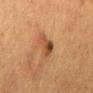Background:
The tile uses cross-polarized illumination. This image is a 15 mm lesion crop taken from a total-body photograph. An algorithmic analysis of the crop reported a lesion area of about 5.5 mm², a shape eccentricity near 0.45, and two-axis asymmetry of about 0.45. It also reported an average lesion color of about L≈39 a*≈20 b*≈30 (CIELAB), a lesion–skin lightness drop of about 10, and a lesion-to-skin contrast of about 8.5 (normalized; higher = more distinct). And it measured a nevus-likeness score of about 85/100 and lesion-presence confidence of about 100/100. Longest diameter approximately 3 mm. A female subject aged around 50. Located on the mid back.